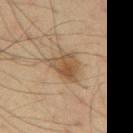This lesion was catalogued during total-body skin photography and was not selected for biopsy. A 15 mm crop from a total-body photograph taken for skin-cancer surveillance. From the arm. Longest diameter approximately 5 mm. A male patient about 35 years old.